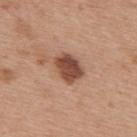{
  "biopsy_status": "not biopsied; imaged during a skin examination",
  "lesion_size": {
    "long_diameter_mm_approx": 3.5
  },
  "site": "upper back",
  "patient": {
    "sex": "female",
    "age_approx": 40
  },
  "automated_metrics": {
    "area_mm2_approx": 8.0,
    "eccentricity": 0.7,
    "shape_asymmetry": 0.15,
    "border_irregularity_0_10": 1.5,
    "color_variation_0_10": 3.5,
    "peripheral_color_asymmetry": 1.0
  },
  "image": {
    "source": "total-body photography crop",
    "field_of_view_mm": 15
  },
  "lighting": "white-light"
}